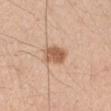Imaged during a routine full-body skin examination; the lesion was not biopsied and no histopathology is available.
The lesion is on the right upper arm.
Captured under white-light illumination.
A 15 mm close-up extracted from a 3D total-body photography capture.
Automated image analysis of the tile measured a nevus-likeness score of about 90/100.
A male patient aged 23 to 27.
Measured at roughly 3 mm in maximum diameter.